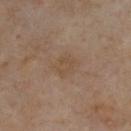The subject is a male about 55 years old. A 15 mm close-up tile from a total-body photography series done for melanoma screening. Located on the front of the torso. Approximately 3 mm at its widest.